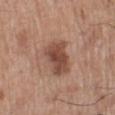Impression:
Part of a total-body skin-imaging series; this lesion was reviewed on a skin check and was not flagged for biopsy.
Acquisition and patient details:
A male patient approximately 70 years of age. The lesion's longest dimension is about 4.5 mm. Located on the left lower leg. The tile uses white-light illumination. A region of skin cropped from a whole-body photographic capture, roughly 15 mm wide. An algorithmic analysis of the crop reported a border-irregularity index near 2.5/10, internal color variation of about 3.5 on a 0–10 scale, and radial color variation of about 1. The analysis additionally found a nevus-likeness score of about 55/100 and lesion-presence confidence of about 100/100.